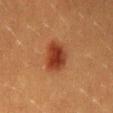Clinical impression: Imaged during a routine full-body skin examination; the lesion was not biopsied and no histopathology is available. Image and clinical context: Imaged with cross-polarized lighting. Approximately 4 mm at its widest. A male patient, aged 38 to 42. A 15 mm crop from a total-body photograph taken for skin-cancer surveillance. On the lower back.